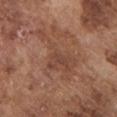Q: Was a biopsy performed?
A: catalogued during a skin exam; not biopsied
Q: Where on the body is the lesion?
A: the chest
Q: What is the imaging modality?
A: ~15 mm crop, total-body skin-cancer survey
Q: Who is the patient?
A: male, aged 73 to 77